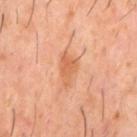• follow-up — total-body-photography surveillance lesion; no biopsy
• anatomic site — the back
• imaging modality — 15 mm crop, total-body photography
• image-analysis metrics — an area of roughly 5.5 mm², an outline eccentricity of about 0.85 (0 = round, 1 = elongated), and two-axis asymmetry of about 0.4; a lesion color around L≈58 a*≈25 b*≈37 in CIELAB, a lesion–skin lightness drop of about 8, and a normalized border contrast of about 6.5; a classifier nevus-likeness of about 0/100 and lesion-presence confidence of about 100/100
• tile lighting — cross-polarized
• subject — male, in their 60s